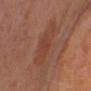Captured during whole-body skin photography for melanoma surveillance; the lesion was not biopsied.
The total-body-photography lesion software estimated a lesion area of about 12 mm², an outline eccentricity of about 0.75 (0 = round, 1 = elongated), and two-axis asymmetry of about 0.25. And it measured an average lesion color of about L≈40 a*≈23 b*≈27 (CIELAB) and a normalized border contrast of about 5. The analysis additionally found a border-irregularity rating of about 3/10, internal color variation of about 2 on a 0–10 scale, and a peripheral color-asymmetry measure near 0.5. It also reported a classifier nevus-likeness of about 15/100 and lesion-presence confidence of about 100/100.
The lesion is on the left thigh.
A close-up tile cropped from a whole-body skin photograph, about 15 mm across.
The lesion's longest dimension is about 4.5 mm.
A female patient aged approximately 50.
Imaged with cross-polarized lighting.